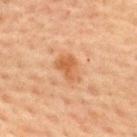biopsy_status: not biopsied; imaged during a skin examination
lighting: cross-polarized
patient:
  sex: male
  age_approx: 60
image:
  source: total-body photography crop
  field_of_view_mm: 15
automated_metrics:
  nevus_likeness_0_100: 70
  lesion_detection_confidence_0_100: 100
site: upper back
lesion_size:
  long_diameter_mm_approx: 3.5